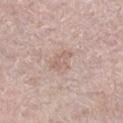This lesion was catalogued during total-body skin photography and was not selected for biopsy. Located on the left leg. A female patient, in their mid- to late 60s. This is a white-light tile. A 15 mm close-up extracted from a 3D total-body photography capture.